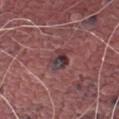Part of a total-body skin-imaging series; this lesion was reviewed on a skin check and was not flagged for biopsy.
The subject is a male aged around 80.
A lesion tile, about 15 mm wide, cut from a 3D total-body photograph.
Located on the right thigh.